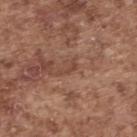No biopsy was performed on this lesion — it was imaged during a full skin examination and was not determined to be concerning.
Cropped from a whole-body photographic skin survey; the tile spans about 15 mm.
On the back.
The tile uses white-light illumination.
A male patient aged 73–77.